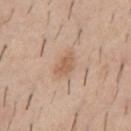Imaged during a routine full-body skin examination; the lesion was not biopsied and no histopathology is available. This image is a 15 mm lesion crop taken from a total-body photograph. The subject is a male aged 38 to 42. From the chest. The recorded lesion diameter is about 3 mm.